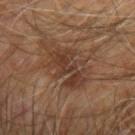Q: What are the patient's age and sex?
A: male, about 60 years old
Q: What is the anatomic site?
A: the left arm
Q: How large is the lesion?
A: about 6 mm
Q: How was this image acquired?
A: 15 mm crop, total-body photography
Q: What lighting was used for the tile?
A: cross-polarized illumination